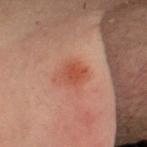Notes:
• notes — imaged on a skin check; not biopsied
• illumination — cross-polarized
• body site — the head or neck
• patient — male, in their 30s
• automated lesion analysis — a footprint of about 4.5 mm², an outline eccentricity of about 0.65 (0 = round, 1 = elongated), and a symmetry-axis asymmetry near 0.2; a mean CIELAB color near L≈40 a*≈25 b*≈27 and roughly 8 lightness units darker than nearby skin; a border-irregularity rating of about 2/10, internal color variation of about 2 on a 0–10 scale, and a peripheral color-asymmetry measure near 1; a detector confidence of about 100 out of 100 that the crop contains a lesion
• lesion diameter — ~2.5 mm (longest diameter)
• imaging modality — total-body-photography crop, ~15 mm field of view